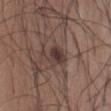Assessment:
The lesion was photographed on a routine skin check and not biopsied; there is no pathology result.
Context:
The lesion is on the abdomen. The patient is a male roughly 25 years of age. A 15 mm crop from a total-body photograph taken for skin-cancer surveillance.